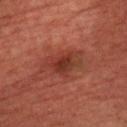notes = imaged on a skin check; not biopsied
anatomic site = the upper back
image source = ~15 mm tile from a whole-body skin photo
patient = male, aged around 65
automated metrics = roughly 7 lightness units darker than nearby skin; a nevus-likeness score of about 65/100
tile lighting = cross-polarized
size = ~5.5 mm (longest diameter)